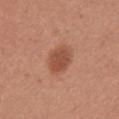Captured during whole-body skin photography for melanoma surveillance; the lesion was not biopsied.
The recorded lesion diameter is about 3 mm.
Automated tile analysis of the lesion measured a mean CIELAB color near L≈50 a*≈25 b*≈32, about 10 CIELAB-L* units darker than the surrounding skin, and a normalized lesion–skin contrast near 7.5. The analysis additionally found a border-irregularity rating of about 1.5/10, a within-lesion color-variation index near 2.5/10, and peripheral color asymmetry of about 1.
Captured under white-light illumination.
The lesion is located on the left upper arm.
Cropped from a whole-body photographic skin survey; the tile spans about 15 mm.
A female patient aged 23–27.